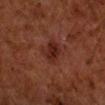The lesion was tiled from a total-body skin photograph and was not biopsied.
Approximately 3 mm at its widest.
A male subject approximately 60 years of age.
This image is a 15 mm lesion crop taken from a total-body photograph.
This is a cross-polarized tile.
Automated tile analysis of the lesion measured border irregularity of about 3 on a 0–10 scale, internal color variation of about 3 on a 0–10 scale, and a peripheral color-asymmetry measure near 1. It also reported a classifier nevus-likeness of about 60/100.
On the right forearm.